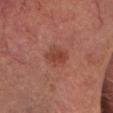Clinical impression:
Recorded during total-body skin imaging; not selected for excision or biopsy.
Context:
On the head or neck. Cropped from a whole-body photographic skin survey; the tile spans about 15 mm. An algorithmic analysis of the crop reported a footprint of about 5 mm², an outline eccentricity of about 0.55 (0 = round, 1 = elongated), and a symmetry-axis asymmetry near 0.25. The analysis additionally found a mean CIELAB color near L≈38 a*≈24 b*≈27, about 7 CIELAB-L* units darker than the surrounding skin, and a normalized border contrast of about 6.5. The software also gave a border-irregularity rating of about 2.5/10, a within-lesion color-variation index near 3/10, and a peripheral color-asymmetry measure near 1. The software also gave a lesion-detection confidence of about 100/100. The lesion's longest dimension is about 2.5 mm. A female patient roughly 60 years of age.